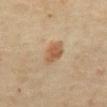This lesion was catalogued during total-body skin photography and was not selected for biopsy.
A 15 mm close-up tile from a total-body photography series done for melanoma screening.
On the abdomen.
A male subject, aged 68 to 72.